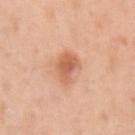notes: total-body-photography surveillance lesion; no biopsy | patient: female, in their mid- to late 40s | image: ~15 mm crop, total-body skin-cancer survey | site: the left upper arm.